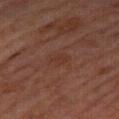Recorded during total-body skin imaging; not selected for excision or biopsy. A male patient aged around 60. A lesion tile, about 15 mm wide, cut from a 3D total-body photograph. The lesion is on the right thigh.